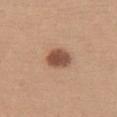Imaged during a routine full-body skin examination; the lesion was not biopsied and no histopathology is available. The lesion is on the chest. A female subject about 20 years old. The lesion's longest dimension is about 3.5 mm. A 15 mm close-up tile from a total-body photography series done for melanoma screening. Imaged with white-light lighting.